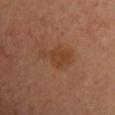This lesion was catalogued during total-body skin photography and was not selected for biopsy.
A region of skin cropped from a whole-body photographic capture, roughly 15 mm wide.
The lesion is located on the chest.
The patient is a female approximately 35 years of age.
Measured at roughly 4.5 mm in maximum diameter.
An algorithmic analysis of the crop reported a mean CIELAB color near L≈40 a*≈21 b*≈32, a lesion–skin lightness drop of about 6, and a normalized border contrast of about 6.5. The analysis additionally found a border-irregularity index near 5/10 and a color-variation rating of about 2/10. And it measured a nevus-likeness score of about 80/100 and lesion-presence confidence of about 100/100.
Imaged with cross-polarized lighting.